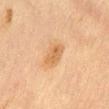workup: no biopsy performed (imaged during a skin exam)
tile lighting: cross-polarized
diameter: about 3 mm
patient: male, about 60 years old
imaging modality: 15 mm crop, total-body photography
site: the abdomen